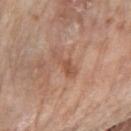Findings:
– follow-up — catalogued during a skin exam; not biopsied
– acquisition — 15 mm crop, total-body photography
– subject — female, aged 83 or over
– image-analysis metrics — a lesion area of about 4.5 mm²; a border-irregularity index near 6.5/10; a classifier nevus-likeness of about 0/100
– size — ≈4 mm
– anatomic site — the right upper arm
– illumination — white-light